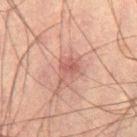On the left leg.
Measured at roughly 3 mm in maximum diameter.
The patient is a male roughly 60 years of age.
A 15 mm close-up tile from a total-body photography series done for melanoma screening.